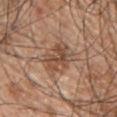Recorded during total-body skin imaging; not selected for excision or biopsy.
Imaged with white-light lighting.
The lesion's longest dimension is about 4.5 mm.
On the back.
A lesion tile, about 15 mm wide, cut from a 3D total-body photograph.
The subject is a male aged 48–52.
The total-body-photography lesion software estimated a lesion area of about 10 mm² and two-axis asymmetry of about 0.25. The analysis additionally found an average lesion color of about L≈49 a*≈20 b*≈30 (CIELAB) and a normalized border contrast of about 7.5. The software also gave a classifier nevus-likeness of about 55/100 and lesion-presence confidence of about 100/100.